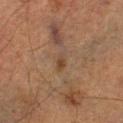follow-up — no biopsy performed (imaged during a skin exam)
patient — male, roughly 75 years of age
diameter — ~3 mm (longest diameter)
location — the left lower leg
TBP lesion metrics — about 5 CIELAB-L* units darker than the surrounding skin and a lesion-to-skin contrast of about 5 (normalized; higher = more distinct); a border-irregularity index near 2/10, a within-lesion color-variation index near 4/10, and radial color variation of about 1.5
acquisition — 15 mm crop, total-body photography